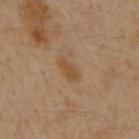Clinical impression: Imaged during a routine full-body skin examination; the lesion was not biopsied and no histopathology is available.